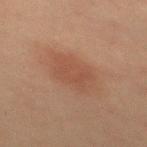Part of a total-body skin-imaging series; this lesion was reviewed on a skin check and was not flagged for biopsy. The patient is a male aged 58–62. Imaged with cross-polarized lighting. A roughly 15 mm field-of-view crop from a total-body skin photograph. From the mid back. Automated image analysis of the tile measured a lesion area of about 13 mm² and a shape eccentricity near 0.75. The analysis additionally found a lesion color around L≈40 a*≈18 b*≈26 in CIELAB, roughly 6 lightness units darker than nearby skin, and a lesion-to-skin contrast of about 5 (normalized; higher = more distinct).